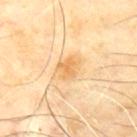biopsy status: no biopsy performed (imaged during a skin exam) | location: the mid back | acquisition: ~15 mm crop, total-body skin-cancer survey | lesion size: about 3 mm | lighting: cross-polarized illumination | patient: male, aged around 60.